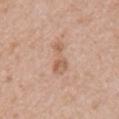Clinical impression:
Recorded during total-body skin imaging; not selected for excision or biopsy.